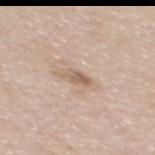biopsy_status: not biopsied; imaged during a skin examination
image:
  source: total-body photography crop
  field_of_view_mm: 15
patient:
  sex: female
  age_approx: 45
site: mid back